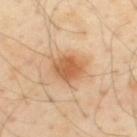Q: Is there a histopathology result?
A: imaged on a skin check; not biopsied